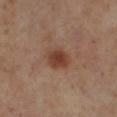This lesion was catalogued during total-body skin photography and was not selected for biopsy. The lesion's longest dimension is about 3 mm. This is a cross-polarized tile. The lesion is located on the left lower leg. A 15 mm crop from a total-body photograph taken for skin-cancer surveillance. A female subject aged around 55.